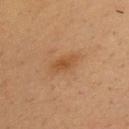Q: Was a biopsy performed?
A: no biopsy performed (imaged during a skin exam)
Q: Who is the patient?
A: male, in their mid-30s
Q: How was this image acquired?
A: total-body-photography crop, ~15 mm field of view
Q: Illumination type?
A: cross-polarized illumination
Q: Automated lesion metrics?
A: an area of roughly 5 mm² and a symmetry-axis asymmetry near 0.25; a lesion color around L≈46 a*≈20 b*≈35 in CIELAB, a lesion–skin lightness drop of about 7, and a normalized border contrast of about 6.5
Q: Where on the body is the lesion?
A: the chest